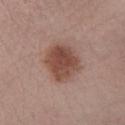workup: imaged on a skin check; not biopsied | subject: male, about 60 years old | acquisition: ~15 mm tile from a whole-body skin photo | location: the leg | lighting: white-light illumination.